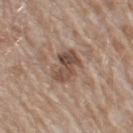{
  "biopsy_status": "not biopsied; imaged during a skin examination",
  "lighting": "white-light",
  "site": "arm",
  "lesion_size": {
    "long_diameter_mm_approx": 4.0
  },
  "patient": {
    "sex": "male",
    "age_approx": 60
  },
  "image": {
    "source": "total-body photography crop",
    "field_of_view_mm": 15
  }
}